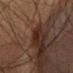Q: Was this lesion biopsied?
A: no biopsy performed (imaged during a skin exam)
Q: Lesion location?
A: the lower back
Q: How was the tile lit?
A: cross-polarized
Q: What is the imaging modality?
A: ~15 mm tile from a whole-body skin photo
Q: Lesion size?
A: about 2.5 mm
Q: What are the patient's age and sex?
A: male, aged 58 to 62